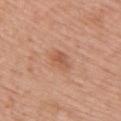From the right upper arm. A roughly 15 mm field-of-view crop from a total-body skin photograph. The lesion-visualizer software estimated an area of roughly 4.5 mm², an outline eccentricity of about 0.75 (0 = round, 1 = elongated), and two-axis asymmetry of about 0.25. And it measured a mean CIELAB color near L≈56 a*≈24 b*≈33 and a lesion–skin lightness drop of about 8. The analysis additionally found a border-irregularity rating of about 2.5/10, internal color variation of about 3 on a 0–10 scale, and radial color variation of about 1. It also reported a detector confidence of about 100 out of 100 that the crop contains a lesion. The tile uses white-light illumination. Measured at roughly 3 mm in maximum diameter. The subject is a female in their mid- to late 60s.